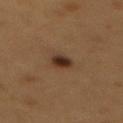{"biopsy_status": "not biopsied; imaged during a skin examination", "image": {"source": "total-body photography crop", "field_of_view_mm": 15}, "patient": {"sex": "male", "age_approx": 55}, "automated_metrics": {"vs_skin_darker_L": 11.0, "vs_skin_contrast_norm": 11.5}, "lesion_size": {"long_diameter_mm_approx": 2.5}, "site": "mid back"}